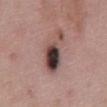Automated image analysis of the tile measured a classifier nevus-likeness of about 0/100 and lesion-presence confidence of about 100/100.
The tile uses white-light illumination.
Located on the abdomen.
Cropped from a total-body skin-imaging series; the visible field is about 15 mm.
The patient is a male aged 48 to 52.
Approximately 6 mm at its widest.
On excision, pathology confirmed an invasive melanoma, superficial spreading type; Breslow depth 0.3 mm, mitotic rate <1/mm².